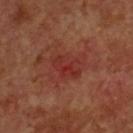Findings:
– follow-up: total-body-photography surveillance lesion; no biopsy
– acquisition: ~15 mm crop, total-body skin-cancer survey
– site: the head or neck
– lesion diameter: ≈4 mm
– TBP lesion metrics: a lesion area of about 7 mm², an eccentricity of roughly 0.8, and a shape-asymmetry score of about 0.25 (0 = symmetric); a mean CIELAB color near L≈32 a*≈29 b*≈27, a lesion–skin lightness drop of about 6, and a normalized border contrast of about 6
– subject: male, roughly 60 years of age